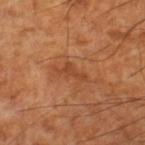Recorded during total-body skin imaging; not selected for excision or biopsy. Located on the leg. The lesion's longest dimension is about 3.5 mm. A male patient, about 60 years old. This image is a 15 mm lesion crop taken from a total-body photograph.